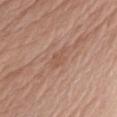Automated tile analysis of the lesion measured an area of roughly 4 mm² and a shape eccentricity near 0.75. It also reported a border-irregularity rating of about 3.5/10, a within-lesion color-variation index near 1/10, and radial color variation of about 0.5.
A female subject, aged 53 to 57.
This image is a 15 mm lesion crop taken from a total-body photograph.
This is a white-light tile.
Measured at roughly 3 mm in maximum diameter.
From the left upper arm.